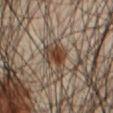Acquisition and patient details: A 15 mm crop from a total-body photograph taken for skin-cancer surveillance. The lesion is on the abdomen. This is a cross-polarized tile. Approximately 5.5 mm at its widest. A male subject aged 43–47.